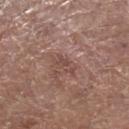| key | value |
|---|---|
| notes | imaged on a skin check; not biopsied |